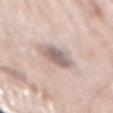biopsy status = imaged on a skin check; not biopsied | imaging modality = ~15 mm tile from a whole-body skin photo | lighting = white-light | body site = the back | subject = male, in their 80s | diameter = about 4 mm.